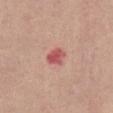{"biopsy_status": "not biopsied; imaged during a skin examination", "patient": {"sex": "female", "age_approx": 65}, "image": {"source": "total-body photography crop", "field_of_view_mm": 15}, "lesion_size": {"long_diameter_mm_approx": 2.5}, "lighting": "white-light", "site": "abdomen"}